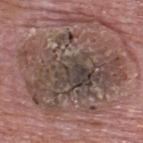notes=no biopsy performed (imaged during a skin exam)
diameter=≈11.5 mm
location=the back
illumination=white-light
image=~15 mm crop, total-body skin-cancer survey
patient=male, aged 73–77